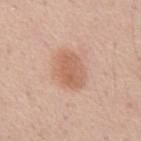No biopsy was performed on this lesion — it was imaged during a full skin examination and was not determined to be concerning. A male patient in their mid- to late 50s. Imaged with white-light lighting. A roughly 15 mm field-of-view crop from a total-body skin photograph. The lesion is on the abdomen. Approximately 4.5 mm at its widest. The lesion-visualizer software estimated a footprint of about 12 mm², an outline eccentricity of about 0.7 (0 = round, 1 = elongated), and a symmetry-axis asymmetry near 0.15. The software also gave border irregularity of about 1.5 on a 0–10 scale and internal color variation of about 3 on a 0–10 scale.